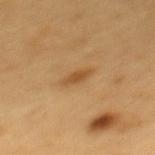Case summary:
- notes — imaged on a skin check; not biopsied
- patient — male, aged 58–62
- anatomic site — the mid back
- image — total-body-photography crop, ~15 mm field of view